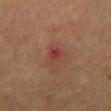This lesion was catalogued during total-body skin photography and was not selected for biopsy. A male subject aged 53–57. A close-up tile cropped from a whole-body skin photograph, about 15 mm across. From the mid back.